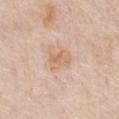Q: Is there a histopathology result?
A: no biopsy performed (imaged during a skin exam)
Q: Who is the patient?
A: male, aged 73 to 77
Q: What lighting was used for the tile?
A: white-light illumination
Q: What is the imaging modality?
A: ~15 mm tile from a whole-body skin photo
Q: Lesion location?
A: the chest
Q: What is the lesion's diameter?
A: about 3 mm
Q: Automated lesion metrics?
A: a mean CIELAB color near L≈66 a*≈18 b*≈34, a lesion–skin lightness drop of about 7, and a normalized border contrast of about 6.5; a border-irregularity index near 2/10, internal color variation of about 3 on a 0–10 scale, and peripheral color asymmetry of about 1